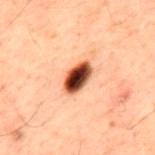No biopsy was performed on this lesion — it was imaged during a full skin examination and was not determined to be concerning. The recorded lesion diameter is about 4 mm. The lesion is located on the back. A male patient, aged around 60. Imaged with cross-polarized lighting. A close-up tile cropped from a whole-body skin photograph, about 15 mm across.